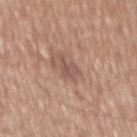follow-up: imaged on a skin check; not biopsied | acquisition: total-body-photography crop, ~15 mm field of view | diameter: ≈3 mm | body site: the mid back | subject: male, roughly 70 years of age | automated lesion analysis: an area of roughly 4 mm² and an outline eccentricity of about 0.7 (0 = round, 1 = elongated).